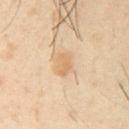Part of a total-body skin-imaging series; this lesion was reviewed on a skin check and was not flagged for biopsy.
A male subject, aged 38 to 42.
The lesion-visualizer software estimated internal color variation of about 1.5 on a 0–10 scale and peripheral color asymmetry of about 0.5.
Captured under cross-polarized illumination.
About 2.5 mm across.
A close-up tile cropped from a whole-body skin photograph, about 15 mm across.
Located on the right upper arm.